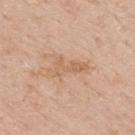biopsy_status: not biopsied; imaged during a skin examination
image:
  source: total-body photography crop
  field_of_view_mm: 15
automated_metrics:
  color_variation_0_10: 2.5
  peripheral_color_asymmetry: 0.5
  lesion_detection_confidence_0_100: 100
lighting: white-light
site: upper back
lesion_size:
  long_diameter_mm_approx: 5.0
patient:
  sex: male
  age_approx: 65